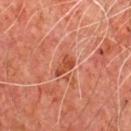biopsy status: catalogued during a skin exam; not biopsied
image source: 15 mm crop, total-body photography
body site: the chest
patient: male, aged 63–67
size: ≈3 mm
automated lesion analysis: a mean CIELAB color near L≈44 a*≈29 b*≈34, about 9 CIELAB-L* units darker than the surrounding skin, and a lesion-to-skin contrast of about 8 (normalized; higher = more distinct); a within-lesion color-variation index near 2/10 and peripheral color asymmetry of about 0.5; a classifier nevus-likeness of about 0/100 and lesion-presence confidence of about 100/100
tile lighting: cross-polarized